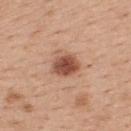{
  "biopsy_status": "not biopsied; imaged during a skin examination",
  "lesion_size": {
    "long_diameter_mm_approx": 3.5
  },
  "lighting": "white-light",
  "image": {
    "source": "total-body photography crop",
    "field_of_view_mm": 15
  },
  "site": "back",
  "patient": {
    "sex": "female",
    "age_approx": 40
  }
}